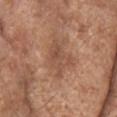Recorded during total-body skin imaging; not selected for excision or biopsy. A male subject, roughly 85 years of age or older. A 15 mm crop from a total-body photograph taken for skin-cancer surveillance. From the head or neck. Measured at roughly 4.5 mm in maximum diameter. The lesion-visualizer software estimated an area of roughly 9 mm² and two-axis asymmetry of about 0.35. Imaged with white-light lighting.